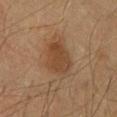The lesion was photographed on a routine skin check and not biopsied; there is no pathology result. The lesion is on the upper back. Captured under cross-polarized illumination. The total-body-photography lesion software estimated an area of roughly 10 mm², a shape eccentricity near 0.75, and a shape-asymmetry score of about 0.2 (0 = symmetric). And it measured a border-irregularity index near 2/10 and a within-lesion color-variation index near 3/10. Approximately 4 mm at its widest. A male subject about 60 years old. A lesion tile, about 15 mm wide, cut from a 3D total-body photograph.